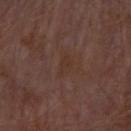Impression: Part of a total-body skin-imaging series; this lesion was reviewed on a skin check and was not flagged for biopsy. Background: A male patient aged 68–72. The lesion is located on the left forearm. An algorithmic analysis of the crop reported an average lesion color of about L≈33 a*≈17 b*≈23 (CIELAB) and a normalized border contrast of about 4.5. The analysis additionally found a border-irregularity rating of about 3.5/10, internal color variation of about 1 on a 0–10 scale, and peripheral color asymmetry of about 0.5. Cropped from a total-body skin-imaging series; the visible field is about 15 mm. The lesion's longest dimension is about 3 mm.